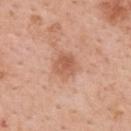<tbp_lesion>
  <biopsy_status>not biopsied; imaged during a skin examination</biopsy_status>
  <lesion_size>
    <long_diameter_mm_approx>3.0</long_diameter_mm_approx>
  </lesion_size>
  <lighting>white-light</lighting>
  <image>
    <source>total-body photography crop</source>
    <field_of_view_mm>15</field_of_view_mm>
  </image>
  <patient>
    <sex>female</sex>
    <age_approx>50</age_approx>
  </patient>
  <site>upper back</site>
</tbp_lesion>